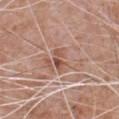| feature | finding |
|---|---|
| follow-up | imaged on a skin check; not biopsied |
| site | the chest |
| illumination | white-light |
| imaging modality | total-body-photography crop, ~15 mm field of view |
| patient | male, aged around 65 |